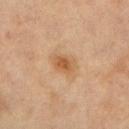Captured during whole-body skin photography for melanoma surveillance; the lesion was not biopsied.